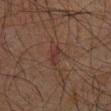follow-up = total-body-photography surveillance lesion; no biopsy
acquisition = ~15 mm crop, total-body skin-cancer survey
diameter = about 4.5 mm
patient = male, aged approximately 60
site = the chest